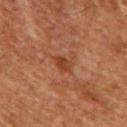  biopsy_status: not biopsied; imaged during a skin examination
  lighting: cross-polarized
  lesion_size:
    long_diameter_mm_approx: 2.5
  image:
    source: total-body photography crop
    field_of_view_mm: 15
  site: front of the torso
  automated_metrics:
    area_mm2_approx: 3.0
    eccentricity: 0.8
    shape_asymmetry: 0.25
  patient:
    sex: male
    age_approx: 60